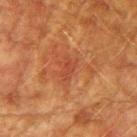{
  "biopsy_status": "not biopsied; imaged during a skin examination",
  "lesion_size": {
    "long_diameter_mm_approx": 3.0
  },
  "site": "right upper arm",
  "patient": {
    "sex": "male",
    "age_approx": 75
  },
  "image": {
    "source": "total-body photography crop",
    "field_of_view_mm": 15
  },
  "lighting": "cross-polarized"
}